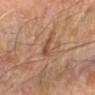notes=imaged on a skin check; not biopsied
location=the left forearm
lesion diameter=about 3 mm
acquisition=15 mm crop, total-body photography
patient=male, aged 68 to 72